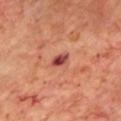| field | value |
|---|---|
| workup | no biopsy performed (imaged during a skin exam) |
| image-analysis metrics | a footprint of about 3 mm² and a symmetry-axis asymmetry near 0.25; a border-irregularity rating of about 2/10, a within-lesion color-variation index near 5/10, and peripheral color asymmetry of about 2; a lesion-detection confidence of about 100/100 |
| image source | total-body-photography crop, ~15 mm field of view |
| site | the chest |
| patient | male, aged 68–72 |
| size | about 2.5 mm |
| illumination | cross-polarized |